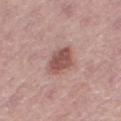workup: imaged on a skin check; not biopsied
site: the right thigh
size: about 3.5 mm
lighting: white-light illumination
patient: female, aged around 50
acquisition: ~15 mm tile from a whole-body skin photo